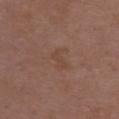Part of a total-body skin-imaging series; this lesion was reviewed on a skin check and was not flagged for biopsy.
About 3 mm across.
From the upper back.
A 15 mm crop from a total-body photograph taken for skin-cancer surveillance.
The lesion-visualizer software estimated an area of roughly 4 mm², an outline eccentricity of about 0.85 (0 = round, 1 = elongated), and a shape-asymmetry score of about 0.35 (0 = symmetric). The software also gave an average lesion color of about L≈44 a*≈19 b*≈26 (CIELAB) and a lesion-to-skin contrast of about 4.5 (normalized; higher = more distinct). The analysis additionally found an automated nevus-likeness rating near 0 out of 100.
This is a white-light tile.
A female subject, approximately 30 years of age.